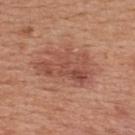No biopsy was performed on this lesion — it was imaged during a full skin examination and was not determined to be concerning.
An algorithmic analysis of the crop reported a border-irregularity index near 5/10, internal color variation of about 5 on a 0–10 scale, and a peripheral color-asymmetry measure near 2. And it measured a nevus-likeness score of about 15/100 and a detector confidence of about 100 out of 100 that the crop contains a lesion.
A 15 mm crop from a total-body photograph taken for skin-cancer surveillance.
On the upper back.
The lesion's longest dimension is about 6.5 mm.
A male patient, aged around 30.